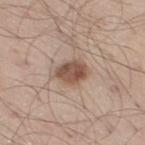| field | value |
|---|---|
| lesion size | ≈3.5 mm |
| patient | male, aged approximately 60 |
| acquisition | total-body-photography crop, ~15 mm field of view |
| site | the right thigh |